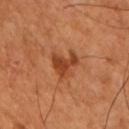Clinical impression:
This lesion was catalogued during total-body skin photography and was not selected for biopsy.
Acquisition and patient details:
On the right upper arm. The lesion-visualizer software estimated a mean CIELAB color near L≈44 a*≈28 b*≈37, about 10 CIELAB-L* units darker than the surrounding skin, and a lesion-to-skin contrast of about 8 (normalized; higher = more distinct). The software also gave internal color variation of about 2.5 on a 0–10 scale and peripheral color asymmetry of about 0.5. A lesion tile, about 15 mm wide, cut from a 3D total-body photograph. A male patient approximately 50 years of age.